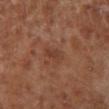<record>
<biopsy_status>not biopsied; imaged during a skin examination</biopsy_status>
<patient>
  <sex>male</sex>
  <age_approx>70</age_approx>
</patient>
<image>
  <source>total-body photography crop</source>
  <field_of_view_mm>15</field_of_view_mm>
</image>
<site>left lower leg</site>
<lesion_size>
  <long_diameter_mm_approx>3.0</long_diameter_mm_approx>
</lesion_size>
</record>